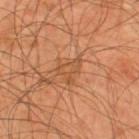imaging modality: ~15 mm tile from a whole-body skin photo | location: the upper back | subject: male, aged approximately 45 | illumination: cross-polarized | diameter: ~3.5 mm (longest diameter) | automated lesion analysis: a footprint of about 5 mm², an outline eccentricity of about 0.7 (0 = round, 1 = elongated), and two-axis asymmetry of about 0.6; border irregularity of about 6.5 on a 0–10 scale, internal color variation of about 2 on a 0–10 scale, and a peripheral color-asymmetry measure near 0.5.